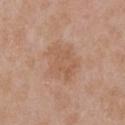notes=imaged on a skin check; not biopsied | lighting=white-light illumination | body site=the front of the torso | TBP lesion metrics=a shape eccentricity near 0.55; an average lesion color of about L≈58 a*≈19 b*≈31 (CIELAB) and about 6 CIELAB-L* units darker than the surrounding skin | subject=female, about 40 years old | image=~15 mm tile from a whole-body skin photo | lesion diameter=≈5.5 mm.